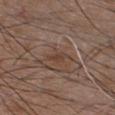The lesion was photographed on a routine skin check and not biopsied; there is no pathology result. The total-body-photography lesion software estimated a lesion area of about 3 mm², an eccentricity of roughly 0.9, and a symmetry-axis asymmetry near 0.35. And it measured a normalized border contrast of about 6. And it measured a border-irregularity index near 4/10, internal color variation of about 0 on a 0–10 scale, and radial color variation of about 0. It also reported a classifier nevus-likeness of about 0/100 and a detector confidence of about 65 out of 100 that the crop contains a lesion. About 3 mm across. The lesion is on the chest. A roughly 15 mm field-of-view crop from a total-body skin photograph. A male patient, about 45 years old.